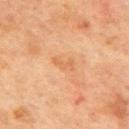The lesion was tiled from a total-body skin photograph and was not biopsied.
The patient is a male approximately 70 years of age.
The lesion is on the mid back.
Automated image analysis of the tile measured a lesion color around L≈52 a*≈22 b*≈34 in CIELAB, roughly 6 lightness units darker than nearby skin, and a normalized lesion–skin contrast near 4.5. The analysis additionally found a border-irregularity rating of about 7/10, internal color variation of about 0 on a 0–10 scale, and a peripheral color-asymmetry measure near 0. The software also gave a classifier nevus-likeness of about 0/100 and a detector confidence of about 100 out of 100 that the crop contains a lesion.
A roughly 15 mm field-of-view crop from a total-body skin photograph.
Imaged with cross-polarized lighting.
The lesion's longest dimension is about 2.5 mm.